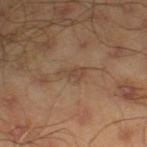Impression:
No biopsy was performed on this lesion — it was imaged during a full skin examination and was not determined to be concerning.